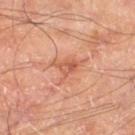notes: no biopsy performed (imaged during a skin exam)
TBP lesion metrics: an area of roughly 5.5 mm², an eccentricity of roughly 0.65, and a shape-asymmetry score of about 0.5 (0 = symmetric); border irregularity of about 6 on a 0–10 scale and a peripheral color-asymmetry measure near 2; a classifier nevus-likeness of about 0/100
lesion diameter: ~3.5 mm (longest diameter)
acquisition: 15 mm crop, total-body photography
subject: male, aged approximately 70
tile lighting: cross-polarized illumination
site: the left thigh